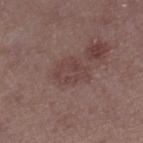Assessment: Part of a total-body skin-imaging series; this lesion was reviewed on a skin check and was not flagged for biopsy. Image and clinical context: Approximately 4 mm at its widest. Automated image analysis of the tile measured a lesion color around L≈42 a*≈19 b*≈20 in CIELAB, roughly 6 lightness units darker than nearby skin, and a normalized border contrast of about 5.5. The analysis additionally found an automated nevus-likeness rating near 0 out of 100. The lesion is located on the right lower leg. Cropped from a whole-body photographic skin survey; the tile spans about 15 mm. The tile uses white-light illumination. A female patient in their 40s.